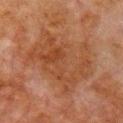{"biopsy_status": "not biopsied; imaged during a skin examination", "lighting": "cross-polarized", "lesion_size": {"long_diameter_mm_approx": 8.0}, "image": {"source": "total-body photography crop", "field_of_view_mm": 15}, "patient": {"sex": "male", "age_approx": 80}, "automated_metrics": {"area_mm2_approx": 37.0, "eccentricity": 0.65, "cielab_L": 36, "cielab_a": 20, "cielab_b": 29, "nevus_likeness_0_100": 0, "lesion_detection_confidence_0_100": 100}, "site": "chest"}